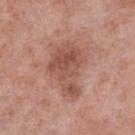Q: How was the tile lit?
A: white-light
Q: Automated lesion metrics?
A: a footprint of about 19 mm², an outline eccentricity of about 0.75 (0 = round, 1 = elongated), and two-axis asymmetry of about 0.4
Q: Patient demographics?
A: male, approximately 55 years of age
Q: Where on the body is the lesion?
A: the left lower leg
Q: Lesion size?
A: ≈6.5 mm
Q: What kind of image is this?
A: 15 mm crop, total-body photography